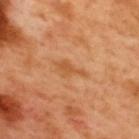follow-up: total-body-photography surveillance lesion; no biopsy | illumination: cross-polarized | image source: total-body-photography crop, ~15 mm field of view | subject: male, aged approximately 50 | lesion size: ≈3.5 mm | body site: the upper back.